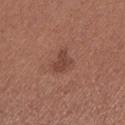Findings:
- notes: total-body-photography surveillance lesion; no biopsy
- lesion diameter: about 2.5 mm
- anatomic site: the left lower leg
- tile lighting: white-light illumination
- imaging modality: total-body-photography crop, ~15 mm field of view
- patient: female, in their mid- to late 50s
- automated lesion analysis: a lesion area of about 4 mm² and a shape-asymmetry score of about 0.45 (0 = symmetric); a mean CIELAB color near L≈43 a*≈23 b*≈26 and about 8 CIELAB-L* units darker than the surrounding skin; a border-irregularity index near 4/10, a within-lesion color-variation index near 1.5/10, and a peripheral color-asymmetry measure near 0.5